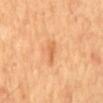No biopsy was performed on this lesion — it was imaged during a full skin examination and was not determined to be concerning. From the mid back. A 15 mm close-up tile from a total-body photography series done for melanoma screening. A male patient, in their mid- to late 60s.